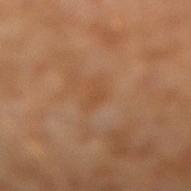Impression:
Captured during whole-body skin photography for melanoma surveillance; the lesion was not biopsied.
Clinical summary:
Captured under cross-polarized illumination. The lesion-visualizer software estimated a footprint of about 3 mm², a shape eccentricity near 0.85, and a symmetry-axis asymmetry near 0.35. The software also gave a lesion-to-skin contrast of about 4.5 (normalized; higher = more distinct). And it measured border irregularity of about 3 on a 0–10 scale and a peripheral color-asymmetry measure near 0.5. It also reported a detector confidence of about 100 out of 100 that the crop contains a lesion. A region of skin cropped from a whole-body photographic capture, roughly 15 mm wide. A male patient aged 28 to 32. From the left leg.